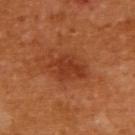biopsy_status: not biopsied; imaged during a skin examination
lesion_size:
  long_diameter_mm_approx: 5.0
automated_metrics:
  area_mm2_approx: 11.0
  eccentricity: 0.8
  shape_asymmetry: 0.15
  vs_skin_darker_L: 8.0
  vs_skin_contrast_norm: 6.5
site: upper back
lighting: cross-polarized
patient:
  sex: female
  age_approx: 55
image:
  source: total-body photography crop
  field_of_view_mm: 15